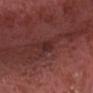Impression:
Part of a total-body skin-imaging series; this lesion was reviewed on a skin check and was not flagged for biopsy.
Background:
Approximately 2.5 mm at its widest. The lesion is on the head or neck. The lesion-visualizer software estimated a mean CIELAB color near L≈28 a*≈23 b*≈20 and a lesion-to-skin contrast of about 6 (normalized; higher = more distinct). The analysis additionally found a border-irregularity index near 2/10 and radial color variation of about 0.5. And it measured a nevus-likeness score of about 0/100 and a detector confidence of about 70 out of 100 that the crop contains a lesion. The tile uses white-light illumination. A 15 mm close-up extracted from a 3D total-body photography capture. A male subject in their mid- to late 70s.